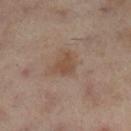Recorded during total-body skin imaging; not selected for excision or biopsy. The subject is a female aged 53–57. From the left leg. This image is a 15 mm lesion crop taken from a total-body photograph. Automated tile analysis of the lesion measured a lesion area of about 5 mm², a shape eccentricity near 0.4, and a symmetry-axis asymmetry near 0.4. And it measured a mean CIELAB color near L≈38 a*≈14 b*≈24 and a lesion-to-skin contrast of about 6.5 (normalized; higher = more distinct). The analysis additionally found a nevus-likeness score of about 5/100 and lesion-presence confidence of about 100/100. Measured at roughly 2.5 mm in maximum diameter. Captured under cross-polarized illumination.